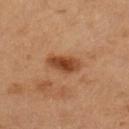biopsy_status: not biopsied; imaged during a skin examination
image:
  source: total-body photography crop
  field_of_view_mm: 15
patient:
  sex: female
  age_approx: 60
lesion_size:
  long_diameter_mm_approx: 4.5
lighting: cross-polarized
site: left lower leg